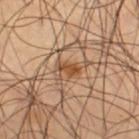Part of a total-body skin-imaging series; this lesion was reviewed on a skin check and was not flagged for biopsy.
A male subject, aged 53 to 57.
This is a cross-polarized tile.
The lesion-visualizer software estimated an area of roughly 3 mm², an outline eccentricity of about 0.85 (0 = round, 1 = elongated), and a symmetry-axis asymmetry near 0.35. The analysis additionally found an automated nevus-likeness rating near 65 out of 100 and a detector confidence of about 100 out of 100 that the crop contains a lesion.
A close-up tile cropped from a whole-body skin photograph, about 15 mm across.
On the right thigh.
About 2.5 mm across.